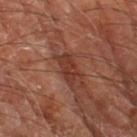follow-up = total-body-photography surveillance lesion; no biopsy | image source = ~15 mm crop, total-body skin-cancer survey | size = ~3.5 mm (longest diameter) | subject = male, roughly 70 years of age | site = the left thigh.